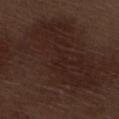This lesion was catalogued during total-body skin photography and was not selected for biopsy. Automated image analysis of the tile measured an eccentricity of roughly 0.9 and a shape-asymmetry score of about 0.5 (0 = symmetric). A close-up tile cropped from a whole-body skin photograph, about 15 mm across. Measured at roughly 22.5 mm in maximum diameter. The lesion is on the leg. A male subject aged 68 to 72.